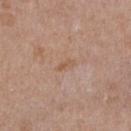Recorded during total-body skin imaging; not selected for excision or biopsy. A lesion tile, about 15 mm wide, cut from a 3D total-body photograph. Automated image analysis of the tile measured a shape eccentricity near 0.85. It also reported a mean CIELAB color near L≈56 a*≈19 b*≈30. And it measured an automated nevus-likeness rating near 0 out of 100 and a lesion-detection confidence of about 100/100. On the chest. Measured at roughly 2.5 mm in maximum diameter. The patient is a female in their mid-30s. Imaged with white-light lighting.